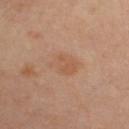automated lesion analysis: an outline eccentricity of about 0.75 (0 = round, 1 = elongated) and a shape-asymmetry score of about 0.25 (0 = symmetric); a classifier nevus-likeness of about 5/100 and a detector confidence of about 100 out of 100 that the crop contains a lesion
diameter: ~4 mm (longest diameter)
body site: the chest
patient: female, aged 48–52
image: total-body-photography crop, ~15 mm field of view
illumination: cross-polarized illumination